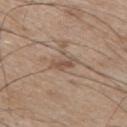notes: no biopsy performed (imaged during a skin exam) | illumination: white-light illumination | lesion diameter: about 3 mm | automated lesion analysis: a footprint of about 4 mm², a shape eccentricity near 0.9, and a shape-asymmetry score of about 0.35 (0 = symmetric); a mean CIELAB color near L≈52 a*≈16 b*≈27; border irregularity of about 4 on a 0–10 scale; a nevus-likeness score of about 0/100 and lesion-presence confidence of about 60/100 | imaging modality: ~15 mm tile from a whole-body skin photo | anatomic site: the mid back | subject: male, aged 73 to 77.